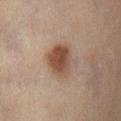  biopsy_status: not biopsied; imaged during a skin examination
  image:
    source: total-body photography crop
    field_of_view_mm: 15
  site: front of the torso
  automated_metrics:
    area_mm2_approx: 9.5
    eccentricity: 0.6
    nevus_likeness_0_100: 100
  lighting: cross-polarized
  lesion_size:
    long_diameter_mm_approx: 4.0
  patient:
    sex: female
    age_approx: 60